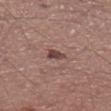  biopsy_status: not biopsied; imaged during a skin examination
  image:
    source: total-body photography crop
    field_of_view_mm: 15
  patient:
    sex: male
    age_approx: 55
  lesion_size:
    long_diameter_mm_approx: 2.5
  site: left lower leg
  lighting: white-light